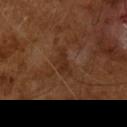biopsy status: catalogued during a skin exam; not biopsied | acquisition: total-body-photography crop, ~15 mm field of view | subject: male, aged around 65 | TBP lesion metrics: a footprint of about 4.5 mm², an eccentricity of roughly 0.85, and a shape-asymmetry score of about 0.45 (0 = symmetric); a mean CIELAB color near L≈28 a*≈19 b*≈27, a lesion–skin lightness drop of about 6, and a normalized lesion–skin contrast near 6 | lesion diameter: about 3.5 mm | lighting: cross-polarized.